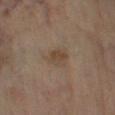| key | value |
|---|---|
| patient | male, aged 68 to 72 |
| size | ~3 mm (longest diameter) |
| site | the right lower leg |
| lighting | cross-polarized illumination |
| image source | ~15 mm tile from a whole-body skin photo |